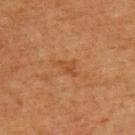Impression: Imaged during a routine full-body skin examination; the lesion was not biopsied and no histopathology is available.